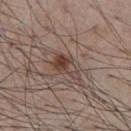Assessment:
Recorded during total-body skin imaging; not selected for excision or biopsy.
Background:
The tile uses white-light illumination. Automated tile analysis of the lesion measured a lesion–skin lightness drop of about 9 and a normalized lesion–skin contrast near 7.5. It also reported border irregularity of about 3.5 on a 0–10 scale and a within-lesion color-variation index near 8/10. The software also gave a classifier nevus-likeness of about 85/100. About 4 mm across. A male patient in their mid- to late 50s. A 15 mm crop from a total-body photograph taken for skin-cancer surveillance. From the chest.